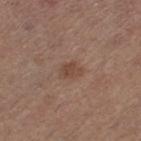Captured during whole-body skin photography for melanoma surveillance; the lesion was not biopsied. A female subject in their mid- to late 40s. Cropped from a total-body skin-imaging series; the visible field is about 15 mm. On the left thigh. Approximately 2.5 mm at its widest. Imaged with white-light lighting.